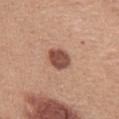Q: Was a biopsy performed?
A: no biopsy performed (imaged during a skin exam)
Q: What is the anatomic site?
A: the left thigh
Q: How large is the lesion?
A: ~3 mm (longest diameter)
Q: What is the imaging modality?
A: ~15 mm crop, total-body skin-cancer survey
Q: Who is the patient?
A: female, approximately 55 years of age
Q: Illumination type?
A: white-light